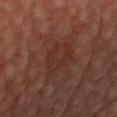<case>
  <biopsy_status>not biopsied; imaged during a skin examination</biopsy_status>
  <patient>
    <age_approx>55</age_approx>
  </patient>
  <lesion_size>
    <long_diameter_mm_approx>5.0</long_diameter_mm_approx>
  </lesion_size>
  <site>front of the torso</site>
  <automated_metrics>
    <cielab_L>30</cielab_L>
    <cielab_a>21</cielab_a>
    <cielab_b>23</cielab_b>
    <vs_skin_darker_L>5.0</vs_skin_darker_L>
    <vs_skin_contrast_norm>5.0</vs_skin_contrast_norm>
    <border_irregularity_0_10>4.0</border_irregularity_0_10>
    <color_variation_0_10>3.0</color_variation_0_10>
    <peripheral_color_asymmetry>1.0</peripheral_color_asymmetry>
  </automated_metrics>
  <image>
    <source>total-body photography crop</source>
    <field_of_view_mm>15</field_of_view_mm>
  </image>
</case>